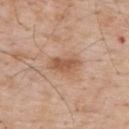| field | value |
|---|---|
| follow-up | no biopsy performed (imaged during a skin exam) |
| patient | male, aged 58 to 62 |
| body site | the upper back |
| TBP lesion metrics | an automated nevus-likeness rating near 45 out of 100 |
| lesion diameter | about 4 mm |
| image source | ~15 mm crop, total-body skin-cancer survey |